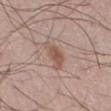Impression:
Imaged during a routine full-body skin examination; the lesion was not biopsied and no histopathology is available.
Clinical summary:
A male subject aged approximately 55. A 15 mm close-up extracted from a 3D total-body photography capture.